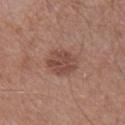Impression: Imaged during a routine full-body skin examination; the lesion was not biopsied and no histopathology is available. Image and clinical context: From the right lower leg. A male patient, aged 63–67. A 15 mm close-up tile from a total-body photography series done for melanoma screening. The tile uses white-light illumination. Longest diameter approximately 3.5 mm.